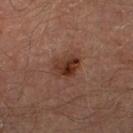The recorded lesion diameter is about 3.5 mm. A roughly 15 mm field-of-view crop from a total-body skin photograph. On the right lower leg. A male subject, aged approximately 65. This is a cross-polarized tile.